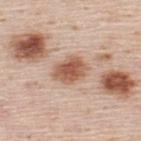This lesion was catalogued during total-body skin photography and was not selected for biopsy.
A male patient, aged approximately 45.
Located on the upper back.
A roughly 15 mm field-of-view crop from a total-body skin photograph.